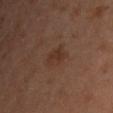Q: Was a biopsy performed?
A: imaged on a skin check; not biopsied
Q: Lesion location?
A: the front of the torso
Q: What are the patient's age and sex?
A: male, aged around 40
Q: Automated lesion metrics?
A: lesion-presence confidence of about 100/100
Q: What kind of image is this?
A: ~15 mm tile from a whole-body skin photo
Q: How large is the lesion?
A: ≈3 mm